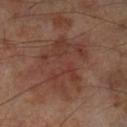- follow-up — catalogued during a skin exam; not biopsied
- site — the left lower leg
- image source — ~15 mm crop, total-body skin-cancer survey
- TBP lesion metrics — a lesion area of about 32 mm², an eccentricity of roughly 0.7, and a shape-asymmetry score of about 0.35 (0 = symmetric); an average lesion color of about L≈37 a*≈20 b*≈24 (CIELAB), a lesion–skin lightness drop of about 6, and a normalized lesion–skin contrast near 5.5; a border-irregularity rating of about 4.5/10 and internal color variation of about 4.5 on a 0–10 scale
- lesion size — ≈8 mm
- patient — male, aged around 70